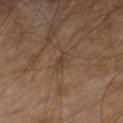Q: Is there a histopathology result?
A: no biopsy performed (imaged during a skin exam)
Q: What are the patient's age and sex?
A: roughly 55 years of age
Q: What is the imaging modality?
A: ~15 mm crop, total-body skin-cancer survey
Q: Lesion location?
A: the right lower leg